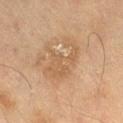Case summary:
• image-analysis metrics · an average lesion color of about L≈47 a*≈15 b*≈30 (CIELAB) and a normalized lesion–skin contrast near 5; a lesion-detection confidence of about 100/100
• anatomic site · the right thigh
• lesion diameter · about 6 mm
• subject · male, in their 70s
• lighting · cross-polarized illumination
• image · ~15 mm crop, total-body skin-cancer survey